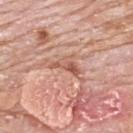The lesion was photographed on a routine skin check and not biopsied; there is no pathology result.
From the upper back.
A region of skin cropped from a whole-body photographic capture, roughly 15 mm wide.
This is a white-light tile.
A male patient, in their 80s.
About 3.5 mm across.
An algorithmic analysis of the crop reported a lesion area of about 4 mm² and a symmetry-axis asymmetry near 0.5. The software also gave an average lesion color of about L≈57 a*≈24 b*≈30 (CIELAB), about 10 CIELAB-L* units darker than the surrounding skin, and a normalized lesion–skin contrast near 6.5. The software also gave a color-variation rating of about 5.5/10 and peripheral color asymmetry of about 2.